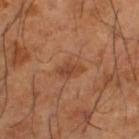No biopsy was performed on this lesion — it was imaged during a full skin examination and was not determined to be concerning.
From the left thigh.
A 15 mm close-up tile from a total-body photography series done for melanoma screening.
About 3 mm across.
The lesion-visualizer software estimated an average lesion color of about L≈44 a*≈24 b*≈34 (CIELAB), roughly 8 lightness units darker than nearby skin, and a normalized lesion–skin contrast near 6.5. It also reported a within-lesion color-variation index near 1.5/10 and peripheral color asymmetry of about 0.5. The analysis additionally found a nevus-likeness score of about 20/100 and a detector confidence of about 100 out of 100 that the crop contains a lesion.
The subject is a male aged around 65.
Captured under cross-polarized illumination.